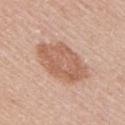{
  "biopsy_status": "not biopsied; imaged during a skin examination",
  "lesion_size": {
    "long_diameter_mm_approx": 6.5
  },
  "image": {
    "source": "total-body photography crop",
    "field_of_view_mm": 15
  },
  "lighting": "white-light",
  "patient": {
    "sex": "female",
    "age_approx": 50
  },
  "automated_metrics": {
    "area_mm2_approx": 22.0,
    "eccentricity": 0.7,
    "shape_asymmetry": 0.15,
    "border_irregularity_0_10": 2.0,
    "color_variation_0_10": 3.5,
    "peripheral_color_asymmetry": 1.0,
    "nevus_likeness_0_100": 10,
    "lesion_detection_confidence_0_100": 100
  },
  "site": "right upper arm"
}